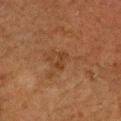Background:
Captured under cross-polarized illumination. A close-up tile cropped from a whole-body skin photograph, about 15 mm across. A male patient, roughly 50 years of age. From the head or neck.